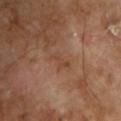| key | value |
|---|---|
| follow-up | no biopsy performed (imaged during a skin exam) |
| patient | male, approximately 70 years of age |
| image source | ~15 mm crop, total-body skin-cancer survey |
| tile lighting | cross-polarized illumination |
| location | the left upper arm |